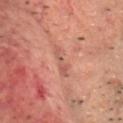imaging modality — total-body-photography crop, ~15 mm field of view
image-analysis metrics — a lesion area of about 4.5 mm² and an outline eccentricity of about 0.95 (0 = round, 1 = elongated); a normalized lesion–skin contrast near 5
anatomic site — the head or neck
patient — male, roughly 70 years of age
tile lighting — cross-polarized illumination
lesion size — ≈4 mm